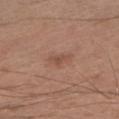Clinical impression: No biopsy was performed on this lesion — it was imaged during a full skin examination and was not determined to be concerning. Context: The lesion is on the right upper arm. The patient is a male aged 58 to 62. Imaged with white-light lighting. This image is a 15 mm lesion crop taken from a total-body photograph.